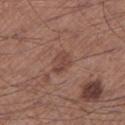No biopsy was performed on this lesion — it was imaged during a full skin examination and was not determined to be concerning. The lesion is on the leg. Captured under white-light illumination. The lesion-visualizer software estimated an area of roughly 4 mm² and an outline eccentricity of about 0.7 (0 = round, 1 = elongated). And it measured border irregularity of about 3 on a 0–10 scale, a within-lesion color-variation index near 2/10, and peripheral color asymmetry of about 1. Measured at roughly 2.5 mm in maximum diameter. This image is a 15 mm lesion crop taken from a total-body photograph. A male patient, aged 58–62.